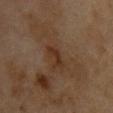• notes — catalogued during a skin exam; not biopsied
• lighting — cross-polarized
• lesion size — about 3 mm
• location — the chest
• patient — female, approximately 60 years of age
• acquisition — ~15 mm tile from a whole-body skin photo
• TBP lesion metrics — a lesion area of about 4 mm², a shape eccentricity near 0.9, and two-axis asymmetry of about 0.35; a lesion–skin lightness drop of about 6 and a normalized border contrast of about 7; a color-variation rating of about 1/10 and peripheral color asymmetry of about 0.5; a nevus-likeness score of about 5/100 and a lesion-detection confidence of about 100/100